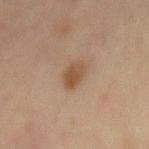Findings:
- follow-up — no biopsy performed (imaged during a skin exam)
- acquisition — 15 mm crop, total-body photography
- subject — male, approximately 45 years of age
- location — the back